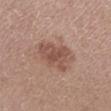| field | value |
|---|---|
| workup | catalogued during a skin exam; not biopsied |
| anatomic site | the left lower leg |
| patient | male, aged 53–57 |
| diameter | ≈5.5 mm |
| image source | total-body-photography crop, ~15 mm field of view |
| lighting | white-light |
| automated lesion analysis | an automated nevus-likeness rating near 25 out of 100 and a detector confidence of about 100 out of 100 that the crop contains a lesion |